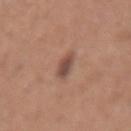The patient is a male about 50 years old. The lesion is located on the right forearm. A 15 mm close-up tile from a total-body photography series done for melanoma screening. Imaged with white-light lighting. The lesion's longest dimension is about 2.5 mm.